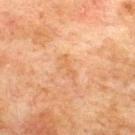biopsy status: imaged on a skin check; not biopsied
patient: male, roughly 75 years of age
body site: the upper back
automated metrics: an average lesion color of about L≈54 a*≈21 b*≈35 (CIELAB) and a lesion-to-skin contrast of about 4.5 (normalized; higher = more distinct)
image: ~15 mm crop, total-body skin-cancer survey